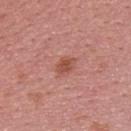follow-up — imaged on a skin check; not biopsied | patient — male, about 25 years old | imaging modality — ~15 mm tile from a whole-body skin photo | body site — the upper back.